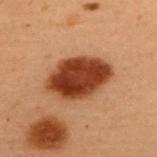The subject is a female aged approximately 50. Cropped from a total-body skin-imaging series; the visible field is about 15 mm. An algorithmic analysis of the crop reported an area of roughly 23 mm², an eccentricity of roughly 0.75, and two-axis asymmetry of about 0.1. It also reported about 18 CIELAB-L* units darker than the surrounding skin and a normalized lesion–skin contrast near 14. From the back.